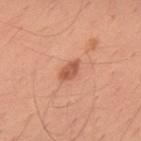Recorded during total-body skin imaging; not selected for excision or biopsy. A male subject roughly 30 years of age. Captured under white-light illumination. A 15 mm close-up extracted from a 3D total-body photography capture. Automated image analysis of the tile measured an area of roughly 3.5 mm², an eccentricity of roughly 0.8, and a symmetry-axis asymmetry near 0.25. On the upper back. Approximately 3 mm at its widest.